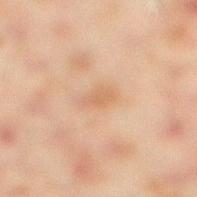{
  "biopsy_status": "not biopsied; imaged during a skin examination",
  "patient": {
    "sex": "male",
    "age_approx": 45
  },
  "site": "right lower leg",
  "lesion_size": {
    "long_diameter_mm_approx": 3.0
  },
  "image": {
    "source": "total-body photography crop",
    "field_of_view_mm": 15
  },
  "lighting": "cross-polarized",
  "automated_metrics": {
    "area_mm2_approx": 4.0,
    "eccentricity": 0.85
  }
}